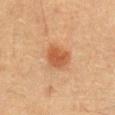follow-up: no biopsy performed (imaged during a skin exam); tile lighting: cross-polarized; patient: male, roughly 50 years of age; anatomic site: the chest; imaging modality: ~15 mm crop, total-body skin-cancer survey.